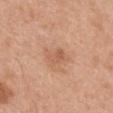{"biopsy_status": "not biopsied; imaged during a skin examination", "image": {"source": "total-body photography crop", "field_of_view_mm": 15}, "lighting": "white-light", "automated_metrics": {"vs_skin_darker_L": 8.0, "vs_skin_contrast_norm": 5.0}, "patient": {"sex": "male", "age_approx": 30}, "site": "mid back"}